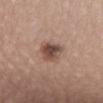biopsy status: no biopsy performed (imaged during a skin exam) | subject: male, in their mid-40s | image: total-body-photography crop, ~15 mm field of view | body site: the lower back.